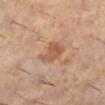automated_metrics:
  area_mm2_approx: 7.0
  eccentricity: 0.6
  cielab_L: 57
  cielab_a: 22
  cielab_b: 34
  vs_skin_contrast_norm: 7.0
patient:
  sex: female
  age_approx: 60
lesion_size:
  long_diameter_mm_approx: 3.5
image:
  source: total-body photography crop
  field_of_view_mm: 15
site: leg